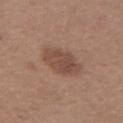Captured during whole-body skin photography for melanoma surveillance; the lesion was not biopsied. Located on the left forearm. The patient is a female about 30 years old. A roughly 15 mm field-of-view crop from a total-body skin photograph. The tile uses white-light illumination. The recorded lesion diameter is about 4 mm.